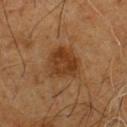Clinical impression:
Part of a total-body skin-imaging series; this lesion was reviewed on a skin check and was not flagged for biopsy.
Background:
The subject is a male roughly 60 years of age. From the chest. A 15 mm crop from a total-body photograph taken for skin-cancer surveillance.